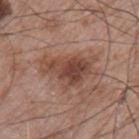biopsy status: catalogued during a skin exam; not biopsied
automated metrics: a footprint of about 16 mm² and an eccentricity of roughly 0.8; border irregularity of about 5.5 on a 0–10 scale and peripheral color asymmetry of about 1.5; a detector confidence of about 100 out of 100 that the crop contains a lesion
illumination: white-light
lesion size: ~6.5 mm (longest diameter)
acquisition: ~15 mm crop, total-body skin-cancer survey
subject: male, aged approximately 65
anatomic site: the left upper arm